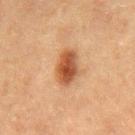The lesion was photographed on a routine skin check and not biopsied; there is no pathology result.
From the mid back.
A close-up tile cropped from a whole-body skin photograph, about 15 mm across.
The subject is a male aged 73 to 77.